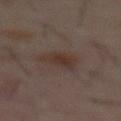follow-up: catalogued during a skin exam; not biopsied
anatomic site: the abdomen
image: ~15 mm crop, total-body skin-cancer survey
TBP lesion metrics: an area of roughly 7 mm² and a symmetry-axis asymmetry near 0.35; a normalized border contrast of about 7; a border-irregularity rating of about 3.5/10, a within-lesion color-variation index near 2.5/10, and a peripheral color-asymmetry measure near 1; a lesion-detection confidence of about 100/100
patient: male, aged 58 to 62
tile lighting: cross-polarized illumination
lesion size: about 4 mm